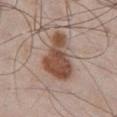| key | value |
|---|---|
| biopsy status | no biopsy performed (imaged during a skin exam) |
| patient | male, aged 53 to 57 |
| anatomic site | the chest |
| image | total-body-photography crop, ~15 mm field of view |